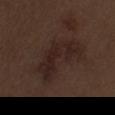No biopsy was performed on this lesion — it was imaged during a full skin examination and was not determined to be concerning. Captured under white-light illumination. A male subject, aged around 70. A 15 mm close-up tile from a total-body photography series done for melanoma screening. The lesion is on the left upper arm. An algorithmic analysis of the crop reported a lesion color around L≈22 a*≈15 b*≈18 in CIELAB, a lesion–skin lightness drop of about 6, and a lesion-to-skin contrast of about 8 (normalized; higher = more distinct). The software also gave radial color variation of about 1.